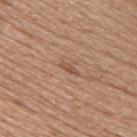<case>
  <biopsy_status>not biopsied; imaged during a skin examination</biopsy_status>
  <patient>
    <sex>female</sex>
    <age_approx>50</age_approx>
  </patient>
  <lighting>white-light</lighting>
  <image>
    <source>total-body photography crop</source>
    <field_of_view_mm>15</field_of_view_mm>
  </image>
  <lesion_size>
    <long_diameter_mm_approx>2.5</long_diameter_mm_approx>
  </lesion_size>
  <site>upper back</site>
</case>